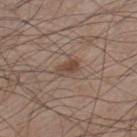notes=imaged on a skin check; not biopsied
patient=male, aged around 60
size=about 2.5 mm
location=the right lower leg
lighting=white-light illumination
image source=total-body-photography crop, ~15 mm field of view